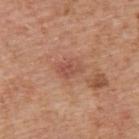The lesion was photographed on a routine skin check and not biopsied; there is no pathology result.
A male subject aged 63 to 67.
This is a white-light tile.
A lesion tile, about 15 mm wide, cut from a 3D total-body photograph.
From the upper back.
Measured at roughly 3 mm in maximum diameter.